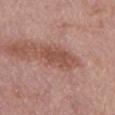follow-up = total-body-photography surveillance lesion; no biopsy
imaging modality = 15 mm crop, total-body photography
anatomic site = the abdomen
patient = male, about 75 years old